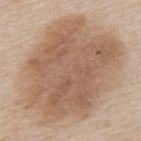Assessment:
Imaged during a routine full-body skin examination; the lesion was not biopsied and no histopathology is available.
Acquisition and patient details:
A male patient approximately 65 years of age. A roughly 15 mm field-of-view crop from a total-body skin photograph. The lesion is on the mid back.